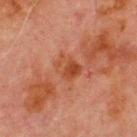{
  "biopsy_status": "not biopsied; imaged during a skin examination",
  "patient": {
    "sex": "male",
    "age_approx": 70
  },
  "site": "chest",
  "image": {
    "source": "total-body photography crop",
    "field_of_view_mm": 15
  },
  "automated_metrics": {
    "area_mm2_approx": 6.0,
    "eccentricity": 0.6,
    "shape_asymmetry": 0.2,
    "cielab_L": 39,
    "cielab_a": 25,
    "cielab_b": 31
  },
  "lighting": "cross-polarized"
}